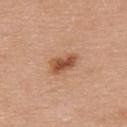The lesion was tiled from a total-body skin photograph and was not biopsied. A 15 mm crop from a total-body photograph taken for skin-cancer surveillance. The subject is a female roughly 65 years of age. Located on the upper back. This is a white-light tile.